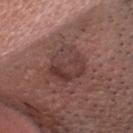| key | value |
|---|---|
| notes | total-body-photography surveillance lesion; no biopsy |
| TBP lesion metrics | a mean CIELAB color near L≈39 a*≈20 b*≈20 and a normalized border contrast of about 6.5; an automated nevus-likeness rating near 15 out of 100 and a lesion-detection confidence of about 100/100 |
| subject | male, aged approximately 35 |
| location | the head or neck |
| tile lighting | white-light |
| imaging modality | 15 mm crop, total-body photography |
| lesion diameter | ≈4.5 mm |